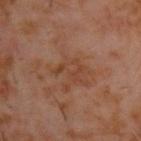Assessment:
No biopsy was performed on this lesion — it was imaged during a full skin examination and was not determined to be concerning.
Acquisition and patient details:
Cropped from a whole-body photographic skin survey; the tile spans about 15 mm. A male subject, roughly 60 years of age. On the upper back.